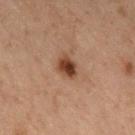Impression:
Captured during whole-body skin photography for melanoma surveillance; the lesion was not biopsied.
Context:
Captured under cross-polarized illumination. A male subject, approximately 70 years of age. Approximately 2.5 mm at its widest. From the abdomen. A roughly 15 mm field-of-view crop from a total-body skin photograph.